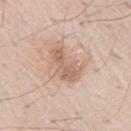biopsy status — total-body-photography surveillance lesion; no biopsy | lesion diameter — about 5 mm | tile lighting — white-light illumination | subject — male, aged 58 to 62 | site — the right upper arm | TBP lesion metrics — a footprint of about 10 mm², an outline eccentricity of about 0.85 (0 = round, 1 = elongated), and two-axis asymmetry of about 0.35; a lesion color around L≈63 a*≈18 b*≈29 in CIELAB and roughly 10 lightness units darker than nearby skin; a nevus-likeness score of about 5/100 and a lesion-detection confidence of about 100/100 | acquisition — total-body-photography crop, ~15 mm field of view.